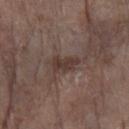workup: imaged on a skin check; not biopsied
subject: female, aged around 85 or older
imaging modality: 15 mm crop, total-body photography
illumination: white-light illumination
automated metrics: a lesion color around L≈36 a*≈15 b*≈20 in CIELAB, a lesion–skin lightness drop of about 9, and a lesion-to-skin contrast of about 8 (normalized; higher = more distinct); a color-variation rating of about 2/10 and radial color variation of about 1; a classifier nevus-likeness of about 0/100 and lesion-presence confidence of about 95/100
location: the arm